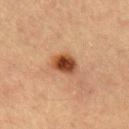{"biopsy_status": "not biopsied; imaged during a skin examination", "site": "left upper arm", "lesion_size": {"long_diameter_mm_approx": 3.5}, "image": {"source": "total-body photography crop", "field_of_view_mm": 15}, "patient": {"sex": "male", "age_approx": 55}}